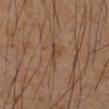The lesion was photographed on a routine skin check and not biopsied; there is no pathology result.
From the left forearm.
The tile uses cross-polarized illumination.
Approximately 3 mm at its widest.
A 15 mm close-up tile from a total-body photography series done for melanoma screening.
A female subject, in their mid- to late 40s.